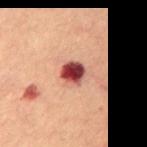follow-up — catalogued during a skin exam; not biopsied
subject — female, aged 58–62
body site — the chest
size — ~3 mm (longest diameter)
tile lighting — cross-polarized
image source — ~15 mm tile from a whole-body skin photo